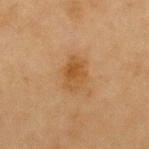Impression:
This lesion was catalogued during total-body skin photography and was not selected for biopsy.
Clinical summary:
The subject is a female aged around 80. Imaged with cross-polarized lighting. The lesion is on the arm. This image is a 15 mm lesion crop taken from a total-body photograph. Longest diameter approximately 4 mm.